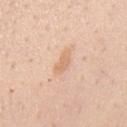Captured during whole-body skin photography for melanoma surveillance; the lesion was not biopsied.
The patient is a male aged 38–42.
The lesion is on the mid back.
Imaged with white-light lighting.
A 15 mm crop from a total-body photograph taken for skin-cancer surveillance.
About 3 mm across.